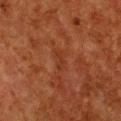No biopsy was performed on this lesion — it was imaged during a full skin examination and was not determined to be concerning. A female subject about 50 years old. This image is a 15 mm lesion crop taken from a total-body photograph. Captured under cross-polarized illumination. The lesion is located on the chest. Longest diameter approximately 2.5 mm. Automated image analysis of the tile measured an area of roughly 2.5 mm², a shape eccentricity near 0.9, and two-axis asymmetry of about 0.3. It also reported a within-lesion color-variation index near 0/10 and a peripheral color-asymmetry measure near 0. And it measured an automated nevus-likeness rating near 0 out of 100 and lesion-presence confidence of about 95/100.